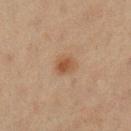The lesion was tiled from a total-body skin photograph and was not biopsied. Located on the leg. The lesion's longest dimension is about 2.5 mm. Cropped from a total-body skin-imaging series; the visible field is about 15 mm. The patient is a female approximately 50 years of age. The tile uses cross-polarized illumination.